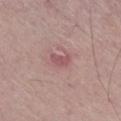Notes:
• biopsy status: imaged on a skin check; not biopsied
• body site: the right lower leg
• illumination: white-light
• image source: ~15 mm tile from a whole-body skin photo
• patient: female, aged 68–72
• diameter: ~2.5 mm (longest diameter)